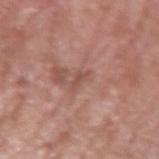| field | value |
|---|---|
| biopsy status | total-body-photography surveillance lesion; no biopsy |
| patient | male, approximately 80 years of age |
| imaging modality | ~15 mm crop, total-body skin-cancer survey |
| site | the left upper arm |